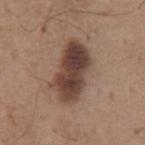Findings:
• lesion diameter · ~6.5 mm (longest diameter)
• tile lighting · white-light illumination
• automated metrics · a footprint of about 20 mm², an eccentricity of roughly 0.85, and a symmetry-axis asymmetry near 0.25; a border-irregularity rating of about 3/10 and a color-variation rating of about 6.5/10; an automated nevus-likeness rating near 85 out of 100
• imaging modality · ~15 mm tile from a whole-body skin photo
• location · the abdomen
• subject · male, aged 63 to 67